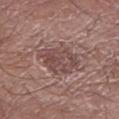This lesion was catalogued during total-body skin photography and was not selected for biopsy.
This is a white-light tile.
The subject is a male aged approximately 55.
On the right forearm.
The lesion's longest dimension is about 5.5 mm.
A 15 mm crop from a total-body photograph taken for skin-cancer surveillance.